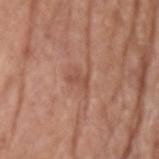Assessment: Recorded during total-body skin imaging; not selected for excision or biopsy.